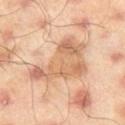{"biopsy_status": "not biopsied; imaged during a skin examination", "lighting": "cross-polarized", "image": {"source": "total-body photography crop", "field_of_view_mm": 15}, "lesion_size": {"long_diameter_mm_approx": 7.5}, "patient": {"sex": "male", "age_approx": 45}, "automated_metrics": {"border_irregularity_0_10": 6.0, "peripheral_color_asymmetry": 1.0, "lesion_detection_confidence_0_100": 95}, "site": "leg"}